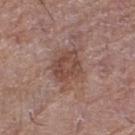follow-up=catalogued during a skin exam; not biopsied
automated metrics=a normalized border contrast of about 7; border irregularity of about 3.5 on a 0–10 scale and a color-variation rating of about 3.5/10; an automated nevus-likeness rating near 30 out of 100 and a detector confidence of about 100 out of 100 that the crop contains a lesion
patient=female, roughly 80 years of age
site=the left thigh
lighting=white-light illumination
imaging modality=~15 mm crop, total-body skin-cancer survey